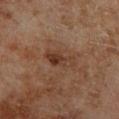Clinical impression: This lesion was catalogued during total-body skin photography and was not selected for biopsy. Background: This image is a 15 mm lesion crop taken from a total-body photograph. Located on the leg. This is a cross-polarized tile. A male patient, roughly 70 years of age. Approximately 4 mm at its widest. The lesion-visualizer software estimated a lesion area of about 6.5 mm², an outline eccentricity of about 0.9 (0 = round, 1 = elongated), and a shape-asymmetry score of about 0.3 (0 = symmetric). And it measured border irregularity of about 4 on a 0–10 scale and internal color variation of about 6 on a 0–10 scale. The analysis additionally found an automated nevus-likeness rating near 75 out of 100 and lesion-presence confidence of about 100/100.